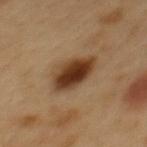Assessment:
The lesion was tiled from a total-body skin photograph and was not biopsied.
Context:
The subject is a male aged approximately 50. Approximately 5 mm at its widest. The lesion is located on the mid back. The tile uses cross-polarized illumination. A roughly 15 mm field-of-view crop from a total-body skin photograph.